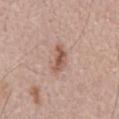Q: Patient demographics?
A: male, approximately 55 years of age
Q: What is the imaging modality?
A: total-body-photography crop, ~15 mm field of view
Q: How was the tile lit?
A: white-light illumination
Q: Automated lesion metrics?
A: an area of roughly 5.5 mm², an outline eccentricity of about 0.85 (0 = round, 1 = elongated), and a symmetry-axis asymmetry near 0.35; a border-irregularity index near 3.5/10 and a peripheral color-asymmetry measure near 1; an automated nevus-likeness rating near 60 out of 100 and a lesion-detection confidence of about 100/100